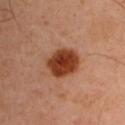Clinical impression:
The lesion was tiled from a total-body skin photograph and was not biopsied.
Clinical summary:
The lesion-visualizer software estimated a mean CIELAB color near L≈38 a*≈26 b*≈32, about 15 CIELAB-L* units darker than the surrounding skin, and a lesion-to-skin contrast of about 12.5 (normalized; higher = more distinct). The analysis additionally found a border-irregularity index near 1.5/10, internal color variation of about 3.5 on a 0–10 scale, and peripheral color asymmetry of about 1. It also reported an automated nevus-likeness rating near 100 out of 100 and lesion-presence confidence of about 100/100. The recorded lesion diameter is about 4.5 mm. The patient is a male aged around 45. From the left upper arm. Captured under cross-polarized illumination. A 15 mm crop from a total-body photograph taken for skin-cancer surveillance.